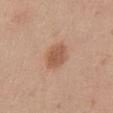The lesion was tiled from a total-body skin photograph and was not biopsied. This image is a 15 mm lesion crop taken from a total-body photograph. The patient is a female roughly 40 years of age. From the abdomen.